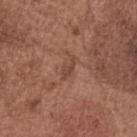Recorded during total-body skin imaging; not selected for excision or biopsy. Approximately 3 mm at its widest. A 15 mm close-up extracted from a 3D total-body photography capture. The lesion-visualizer software estimated an area of roughly 3 mm² and an outline eccentricity of about 0.9 (0 = round, 1 = elongated). The subject is a male approximately 75 years of age. On the head or neck.